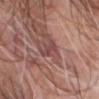{
  "biopsy_status": "not biopsied; imaged during a skin examination",
  "image": {
    "source": "total-body photography crop",
    "field_of_view_mm": 15
  },
  "lesion_size": {
    "long_diameter_mm_approx": 3.0
  },
  "site": "head or neck",
  "patient": {
    "sex": "male",
    "age_approx": 55
  }
}